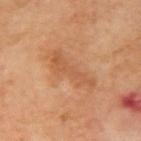The lesion was tiled from a total-body skin photograph and was not biopsied.
A 15 mm close-up tile from a total-body photography series done for melanoma screening.
The lesion is on the right upper arm.
A male patient aged approximately 70.
The recorded lesion diameter is about 6 mm.